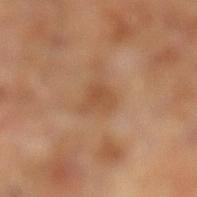Recorded during total-body skin imaging; not selected for excision or biopsy. On the left lower leg. A lesion tile, about 15 mm wide, cut from a 3D total-body photograph. The tile uses cross-polarized illumination. Automated image analysis of the tile measured a footprint of about 5.5 mm² and a shape eccentricity near 0.5. The software also gave an average lesion color of about L≈49 a*≈21 b*≈34 (CIELAB), roughly 7 lightness units darker than nearby skin, and a lesion-to-skin contrast of about 6 (normalized; higher = more distinct). The analysis additionally found a classifier nevus-likeness of about 0/100 and a detector confidence of about 100 out of 100 that the crop contains a lesion. A male subject, roughly 70 years of age. The lesion's longest dimension is about 3 mm.